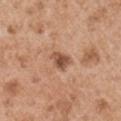Assessment: Recorded during total-body skin imaging; not selected for excision or biopsy. Image and clinical context: A 15 mm close-up tile from a total-body photography series done for melanoma screening. The lesion is located on the right upper arm. The patient is a male about 55 years old. The recorded lesion diameter is about 3 mm. Automated tile analysis of the lesion measured a mean CIELAB color near L≈51 a*≈22 b*≈31, a lesion–skin lightness drop of about 13, and a lesion-to-skin contrast of about 8.5 (normalized; higher = more distinct). The software also gave a within-lesion color-variation index near 3/10.